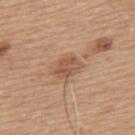Impression:
Recorded during total-body skin imaging; not selected for excision or biopsy.
Clinical summary:
A male subject, aged 63–67. The recorded lesion diameter is about 3.5 mm. A lesion tile, about 15 mm wide, cut from a 3D total-body photograph. Located on the back.